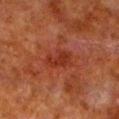{"biopsy_status": "not biopsied; imaged during a skin examination", "image": {"source": "total-body photography crop", "field_of_view_mm": 15}, "patient": {"sex": "male", "age_approx": 80}, "lesion_size": {"long_diameter_mm_approx": 3.5}, "site": "left lower leg", "lighting": "cross-polarized"}